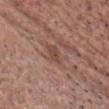Assessment:
Part of a total-body skin-imaging series; this lesion was reviewed on a skin check and was not flagged for biopsy.
Context:
Measured at roughly 2.5 mm in maximum diameter. Imaged with white-light lighting. Cropped from a total-body skin-imaging series; the visible field is about 15 mm. The lesion is on the chest. A male subject, in their mid-50s.